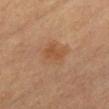The lesion was tiled from a total-body skin photograph and was not biopsied.
The patient is a female in their mid-60s.
A lesion tile, about 15 mm wide, cut from a 3D total-body photograph.
The lesion's longest dimension is about 4 mm.
Located on the mid back.
This is a cross-polarized tile.